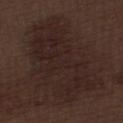This lesion was catalogued during total-body skin photography and was not selected for biopsy. Located on the left thigh. A 15 mm close-up tile from a total-body photography series done for melanoma screening. A male subject, in their 70s.